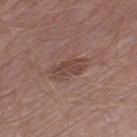Imaged during a routine full-body skin examination; the lesion was not biopsied and no histopathology is available.
Imaged with white-light lighting.
The patient is a male in their mid-50s.
About 4.5 mm across.
The lesion is located on the left thigh.
Automated image analysis of the tile measured a border-irregularity index near 4/10, a within-lesion color-variation index near 2.5/10, and a peripheral color-asymmetry measure near 1.
A region of skin cropped from a whole-body photographic capture, roughly 15 mm wide.